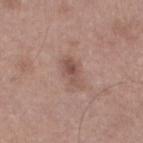The lesion was tiled from a total-body skin photograph and was not biopsied. Automated image analysis of the tile measured an outline eccentricity of about 0.8 (0 = round, 1 = elongated). It also reported a lesion color around L≈51 a*≈19 b*≈24 in CIELAB. The software also gave a border-irregularity rating of about 2.5/10, a within-lesion color-variation index near 4/10, and radial color variation of about 1.5. The analysis additionally found a nevus-likeness score of about 45/100 and lesion-presence confidence of about 100/100. About 3.5 mm across. A roughly 15 mm field-of-view crop from a total-body skin photograph. Imaged with white-light lighting. A male subject in their mid-60s. The lesion is located on the leg.